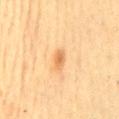Clinical impression:
This lesion was catalogued during total-body skin photography and was not selected for biopsy.
Context:
Measured at roughly 2.5 mm in maximum diameter. From the back. A female patient aged approximately 55. A 15 mm close-up tile from a total-body photography series done for melanoma screening. This is a cross-polarized tile. The lesion-visualizer software estimated an area of roughly 3 mm², an eccentricity of roughly 0.9, and a shape-asymmetry score of about 0.35 (0 = symmetric). The analysis additionally found a mean CIELAB color near L≈58 a*≈20 b*≈39, a lesion–skin lightness drop of about 9, and a normalized border contrast of about 6.5. And it measured a classifier nevus-likeness of about 50/100 and a detector confidence of about 100 out of 100 that the crop contains a lesion.